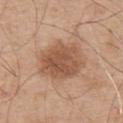The lesion was tiled from a total-body skin photograph and was not biopsied.
This is a white-light tile.
A male patient approximately 55 years of age.
From the upper back.
A 15 mm close-up extracted from a 3D total-body photography capture.
Automated image analysis of the tile measured a nevus-likeness score of about 60/100 and a lesion-detection confidence of about 100/100.